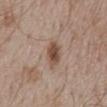  lesion_size:
    long_diameter_mm_approx: 4.0
  image:
    source: total-body photography crop
    field_of_view_mm: 15
  automated_metrics:
    cielab_L: 49
    cielab_a: 18
    cielab_b: 27
    vs_skin_darker_L: 12.0
    vs_skin_contrast_norm: 9.0
    border_irregularity_0_10: 2.0
    nevus_likeness_0_100: 95
    lesion_detection_confidence_0_100: 100
  site: mid back
  patient:
    sex: male
    age_approx: 55
  lighting: white-light